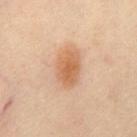– workup — no biopsy performed (imaged during a skin exam)
– site — the chest
– patient — male, in their mid- to late 60s
– image source — total-body-photography crop, ~15 mm field of view